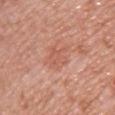Q: What did automated image analysis measure?
A: a footprint of about 8 mm² and an outline eccentricity of about 0.75 (0 = round, 1 = elongated); a border-irregularity rating of about 4.5/10, a color-variation rating of about 2/10, and peripheral color asymmetry of about 1; an automated nevus-likeness rating near 0 out of 100 and a lesion-detection confidence of about 100/100
Q: What is the lesion's diameter?
A: ~4.5 mm (longest diameter)
Q: What is the imaging modality?
A: ~15 mm tile from a whole-body skin photo
Q: How was the tile lit?
A: white-light illumination
Q: Patient demographics?
A: female, approximately 50 years of age
Q: What is the anatomic site?
A: the chest